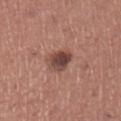{"biopsy_status": "not biopsied; imaged during a skin examination", "site": "left lower leg", "image": {"source": "total-body photography crop", "field_of_view_mm": 15}, "lesion_size": {"long_diameter_mm_approx": 3.0}, "patient": {"sex": "female", "age_approx": 65}}